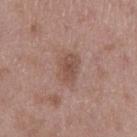patient: male, aged 48 to 52 | image source: ~15 mm crop, total-body skin-cancer survey | site: the left thigh.